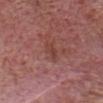{"biopsy_status": "not biopsied; imaged during a skin examination", "patient": {"sex": "male", "age_approx": 75}, "lesion_size": {"long_diameter_mm_approx": 2.5}, "lighting": "white-light", "automated_metrics": {"cielab_L": 42, "cielab_a": 24, "cielab_b": 24, "vs_skin_darker_L": 6.0, "vs_skin_contrast_norm": 5.5, "border_irregularity_0_10": 3.0, "color_variation_0_10": 3.0, "peripheral_color_asymmetry": 1.0, "nevus_likeness_0_100": 0, "lesion_detection_confidence_0_100": 100}, "site": "head or neck", "image": {"source": "total-body photography crop", "field_of_view_mm": 15}}